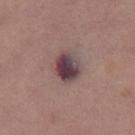The tile uses white-light illumination.
A roughly 15 mm field-of-view crop from a total-body skin photograph.
The lesion-visualizer software estimated an area of roughly 8.5 mm², an outline eccentricity of about 0.5 (0 = round, 1 = elongated), and a symmetry-axis asymmetry near 0.15. And it measured a normalized border contrast of about 12.5. The software also gave a classifier nevus-likeness of about 65/100.
A female subject, roughly 40 years of age.
Longest diameter approximately 3.5 mm.
From the right thigh.